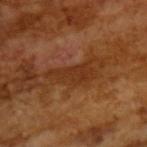Impression: The lesion was photographed on a routine skin check and not biopsied; there is no pathology result. Clinical summary: Approximately 5.5 mm at its widest. A roughly 15 mm field-of-view crop from a total-body skin photograph. A male patient aged 63 to 67. This is a cross-polarized tile.